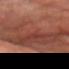Imaged during a routine full-body skin examination; the lesion was not biopsied and no histopathology is available.